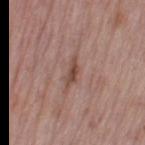subject — female, roughly 50 years of age
site — the leg
acquisition — ~15 mm crop, total-body skin-cancer survey
tile lighting — white-light illumination
lesion size — ≈3.5 mm